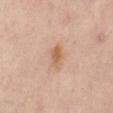Q: Is there a histopathology result?
A: imaged on a skin check; not biopsied
Q: What did automated image analysis measure?
A: a border-irregularity rating of about 2.5/10
Q: How was the tile lit?
A: cross-polarized
Q: Patient demographics?
A: female, roughly 50 years of age
Q: Lesion location?
A: the right thigh
Q: How was this image acquired?
A: 15 mm crop, total-body photography